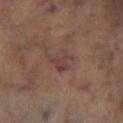follow-up = catalogued during a skin exam; not biopsied
TBP lesion metrics = a lesion area of about 5 mm² and a symmetry-axis asymmetry near 0.55; a border-irregularity index near 7/10, a within-lesion color-variation index near 2.5/10, and radial color variation of about 0.5; an automated nevus-likeness rating near 0 out of 100 and lesion-presence confidence of about 95/100
subject = male, aged around 65
site = the leg
image = total-body-photography crop, ~15 mm field of view
illumination = cross-polarized illumination